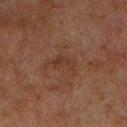Part of a total-body skin-imaging series; this lesion was reviewed on a skin check and was not flagged for biopsy.
The subject is a male in their 60s.
A region of skin cropped from a whole-body photographic capture, roughly 15 mm wide.
The tile uses cross-polarized illumination.
The lesion is on the chest.